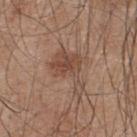Q: Is there a histopathology result?
A: total-body-photography surveillance lesion; no biopsy
Q: What did automated image analysis measure?
A: a lesion area of about 11 mm², a shape eccentricity near 0.8, and a symmetry-axis asymmetry near 0.6; border irregularity of about 7 on a 0–10 scale, a within-lesion color-variation index near 3.5/10, and peripheral color asymmetry of about 1; a classifier nevus-likeness of about 30/100 and a detector confidence of about 100 out of 100 that the crop contains a lesion
Q: Lesion size?
A: about 5 mm
Q: What are the patient's age and sex?
A: male, aged around 45
Q: Illumination type?
A: white-light
Q: What is the imaging modality?
A: ~15 mm tile from a whole-body skin photo
Q: Lesion location?
A: the upper back